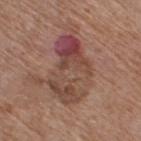Q: What is the anatomic site?
A: the leg
Q: What lighting was used for the tile?
A: white-light illumination
Q: What kind of image is this?
A: 15 mm crop, total-body photography
Q: What is the lesion's diameter?
A: about 8 mm
Q: Who is the patient?
A: female, roughly 75 years of age
Q: Automated lesion metrics?
A: an outline eccentricity of about 0.85 (0 = round, 1 = elongated) and a symmetry-axis asymmetry near 0.35; a lesion color around L≈45 a*≈21 b*≈24 in CIELAB and roughly 9 lightness units darker than nearby skin